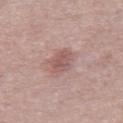Recorded during total-body skin imaging; not selected for excision or biopsy. Approximately 3.5 mm at its widest. A male patient, about 65 years old. A 15 mm crop from a total-body photograph taken for skin-cancer surveillance. From the chest. Automated image analysis of the tile measured an area of roughly 5.5 mm² and two-axis asymmetry of about 0.3. The software also gave a border-irregularity index near 3/10, a within-lesion color-variation index near 3/10, and peripheral color asymmetry of about 1. The tile uses white-light illumination.